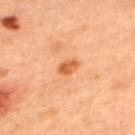Context: The patient is a male in their mid- to late 40s. Measured at roughly 3 mm in maximum diameter. From the upper back. This image is a 15 mm lesion crop taken from a total-body photograph.